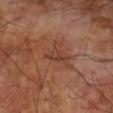  biopsy_status: not biopsied; imaged during a skin examination
  lighting: cross-polarized
  patient:
    sex: male
    age_approx: 70
  site: right forearm
  automated_metrics:
    area_mm2_approx: 3.5
    eccentricity: 0.85
    cielab_L: 39
    cielab_a: 22
    cielab_b: 28
    vs_skin_darker_L: 6.0
    vs_skin_contrast_norm: 5.5
    nevus_likeness_0_100: 0
    lesion_detection_confidence_0_100: 90
  image:
    source: total-body photography crop
    field_of_view_mm: 15
  lesion_size:
    long_diameter_mm_approx: 3.0